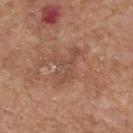notes=no biopsy performed (imaged during a skin exam)
location=the upper back
size=~4.5 mm (longest diameter)
image source=total-body-photography crop, ~15 mm field of view
subject=male, in their mid-60s
image-analysis metrics=lesion-presence confidence of about 100/100